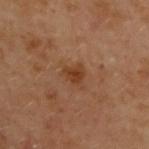workup — no biopsy performed (imaged during a skin exam)
anatomic site — the upper back
automated lesion analysis — an eccentricity of roughly 0.55 and a symmetry-axis asymmetry near 0.4; a lesion color around L≈34 a*≈19 b*≈30 in CIELAB, a lesion–skin lightness drop of about 7, and a normalized border contrast of about 7.5; a border-irregularity index near 3.5/10 and radial color variation of about 0.5
lesion size — ~2.5 mm (longest diameter)
patient — female, aged around 60
tile lighting — cross-polarized illumination
image source — total-body-photography crop, ~15 mm field of view